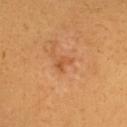Part of a total-body skin-imaging series; this lesion was reviewed on a skin check and was not flagged for biopsy. About 2 mm across. The total-body-photography lesion software estimated a footprint of about 3 mm² and an outline eccentricity of about 0.7 (0 = round, 1 = elongated). The software also gave a border-irregularity index near 3.5/10, a color-variation rating of about 2/10, and peripheral color asymmetry of about 0.5. On the upper back. This is a cross-polarized tile. The subject is a female approximately 25 years of age. A 15 mm crop from a total-body photograph taken for skin-cancer surveillance.